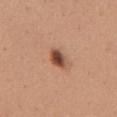Context: The total-body-photography lesion software estimated a lesion area of about 4.5 mm², an eccentricity of roughly 0.65, and two-axis asymmetry of about 0.25. The analysis additionally found a mean CIELAB color near L≈49 a*≈23 b*≈30, a lesion–skin lightness drop of about 16, and a normalized border contrast of about 11. And it measured border irregularity of about 2 on a 0–10 scale, a color-variation rating of about 7.5/10, and radial color variation of about 2. The software also gave a classifier nevus-likeness of about 100/100 and a lesion-detection confidence of about 100/100. The tile uses white-light illumination. This image is a 15 mm lesion crop taken from a total-body photograph. From the chest. Measured at roughly 2.5 mm in maximum diameter. A female subject, aged around 50.